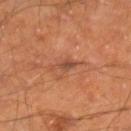Acquisition and patient details:
Imaged with cross-polarized lighting. Automated image analysis of the tile measured an area of roughly 3 mm², a shape eccentricity near 0.9, and a symmetry-axis asymmetry near 0.35. The analysis additionally found a lesion color around L≈42 a*≈22 b*≈29 in CIELAB, about 8 CIELAB-L* units darker than the surrounding skin, and a lesion-to-skin contrast of about 6.5 (normalized; higher = more distinct). And it measured an automated nevus-likeness rating near 0 out of 100. A male patient, aged 63–67. From the right lower leg. A 15 mm close-up extracted from a 3D total-body photography capture.